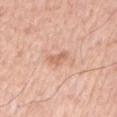No biopsy was performed on this lesion — it was imaged during a full skin examination and was not determined to be concerning. A male subject, roughly 55 years of age. The lesion is on the left upper arm. Cropped from a total-body skin-imaging series; the visible field is about 15 mm.